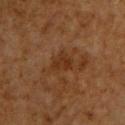Captured during whole-body skin photography for melanoma surveillance; the lesion was not biopsied. The lesion is located on the chest. This image is a 15 mm lesion crop taken from a total-body photograph. The subject is a male about 60 years old.